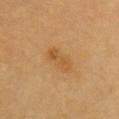Recorded during total-body skin imaging; not selected for excision or biopsy.